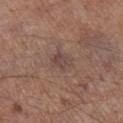workup = no biopsy performed (imaged during a skin exam) | diameter = ~2.5 mm (longest diameter) | patient = male, in their mid-50s | imaging modality = total-body-photography crop, ~15 mm field of view | lighting = white-light illumination | image-analysis metrics = a mean CIELAB color near L≈44 a*≈17 b*≈21 and a normalized border contrast of about 6; a classifier nevus-likeness of about 0/100 and lesion-presence confidence of about 100/100 | anatomic site = the right lower leg.